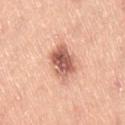The lesion was tiled from a total-body skin photograph and was not biopsied. An algorithmic analysis of the crop reported an area of roughly 11 mm², an outline eccentricity of about 0.75 (0 = round, 1 = elongated), and two-axis asymmetry of about 0.25. A male subject about 50 years old. Approximately 5 mm at its widest. A close-up tile cropped from a whole-body skin photograph, about 15 mm across. The lesion is located on the right thigh.